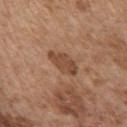Assessment:
The lesion was tiled from a total-body skin photograph and was not biopsied.
Image and clinical context:
The recorded lesion diameter is about 4 mm. From the chest. Captured under white-light illumination. Cropped from a whole-body photographic skin survey; the tile spans about 15 mm. A male subject, in their mid-70s. Automated image analysis of the tile measured border irregularity of about 2.5 on a 0–10 scale, internal color variation of about 1.5 on a 0–10 scale, and a peripheral color-asymmetry measure near 0.5. The software also gave an automated nevus-likeness rating near 20 out of 100 and a lesion-detection confidence of about 100/100.